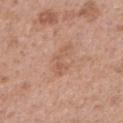Notes:
– workup: total-body-photography surveillance lesion; no biopsy
– acquisition: 15 mm crop, total-body photography
– automated lesion analysis: an area of roughly 4 mm², a shape eccentricity near 0.95, and two-axis asymmetry of about 0.55; a lesion color around L≈57 a*≈22 b*≈30 in CIELAB and about 7 CIELAB-L* units darker than the surrounding skin; a border-irregularity rating of about 9/10, a color-variation rating of about 0/10, and radial color variation of about 0
– anatomic site: the right upper arm
– illumination: white-light illumination
– subject: female, about 40 years old
– diameter: ≈4 mm